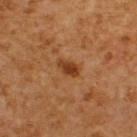Q: What are the patient's age and sex?
A: male, roughly 60 years of age
Q: Lesion size?
A: ≈3 mm
Q: What did automated image analysis measure?
A: a footprint of about 4 mm² and a symmetry-axis asymmetry near 0.25
Q: What kind of image is this?
A: total-body-photography crop, ~15 mm field of view
Q: Where on the body is the lesion?
A: the upper back
Q: Illumination type?
A: cross-polarized illumination